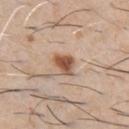Q: Was this lesion biopsied?
A: catalogued during a skin exam; not biopsied
Q: Lesion location?
A: the chest
Q: Who is the patient?
A: male, aged around 60
Q: What kind of image is this?
A: ~15 mm crop, total-body skin-cancer survey